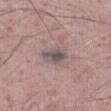follow-up: imaged on a skin check; not biopsied | patient: male, roughly 70 years of age | TBP lesion metrics: border irregularity of about 2.5 on a 0–10 scale, a color-variation rating of about 4.5/10, and peripheral color asymmetry of about 1.5; a classifier nevus-likeness of about 0/100 and a detector confidence of about 75 out of 100 that the crop contains a lesion | site: the abdomen | lesion size: about 4 mm | illumination: white-light | image source: ~15 mm crop, total-body skin-cancer survey.